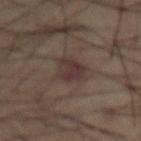A male patient roughly 50 years of age.
A roughly 15 mm field-of-view crop from a total-body skin photograph.
This is a cross-polarized tile.
Longest diameter approximately 3.5 mm.
On the abdomen.